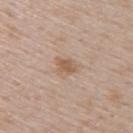{"biopsy_status": "not biopsied; imaged during a skin examination", "site": "upper back", "automated_metrics": {"cielab_L": 57, "cielab_a": 17, "cielab_b": 30, "vs_skin_darker_L": 9.0, "vs_skin_contrast_norm": 6.5, "color_variation_0_10": 2.0, "peripheral_color_asymmetry": 0.5, "nevus_likeness_0_100": 25}, "patient": {"sex": "male", "age_approx": 50}, "image": {"source": "total-body photography crop", "field_of_view_mm": 15}, "lighting": "white-light", "lesion_size": {"long_diameter_mm_approx": 2.5}}